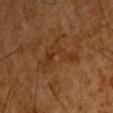Clinical impression:
Imaged during a routine full-body skin examination; the lesion was not biopsied and no histopathology is available.
Image and clinical context:
A region of skin cropped from a whole-body photographic capture, roughly 15 mm wide. A male patient roughly 65 years of age. From the head or neck.